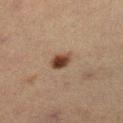Clinical impression: Part of a total-body skin-imaging series; this lesion was reviewed on a skin check and was not flagged for biopsy. Background: The lesion is located on the left lower leg. A female patient about 55 years old. A 15 mm close-up tile from a total-body photography series done for melanoma screening.